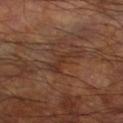Impression: The lesion was photographed on a routine skin check and not biopsied; there is no pathology result. Context: Longest diameter approximately 4 mm. The tile uses cross-polarized illumination. The lesion is on the leg. The lesion-visualizer software estimated a lesion area of about 4.5 mm² and an outline eccentricity of about 0.9 (0 = round, 1 = elongated). The analysis additionally found a border-irregularity rating of about 7/10, a color-variation rating of about 0.5/10, and radial color variation of about 0. It also reported a classifier nevus-likeness of about 0/100. The patient is a male aged 58 to 62. Cropped from a whole-body photographic skin survey; the tile spans about 15 mm.